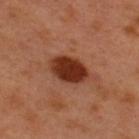• notes · catalogued during a skin exam; not biopsied
• tile lighting · cross-polarized illumination
• body site · the upper back
• lesion size · about 4.5 mm
• image · ~15 mm crop, total-body skin-cancer survey
• patient · male, aged 48 to 52